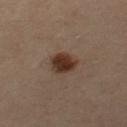The lesion was photographed on a routine skin check and not biopsied; there is no pathology result.
The subject is a female aged 38 to 42.
The recorded lesion diameter is about 3.5 mm.
This is a cross-polarized tile.
The lesion is located on the right leg.
A lesion tile, about 15 mm wide, cut from a 3D total-body photograph.